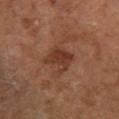This lesion was catalogued during total-body skin photography and was not selected for biopsy. The total-body-photography lesion software estimated an eccentricity of roughly 0.55 and a shape-asymmetry score of about 0.3 (0 = symmetric). It also reported border irregularity of about 3.5 on a 0–10 scale, internal color variation of about 2.5 on a 0–10 scale, and peripheral color asymmetry of about 1. A region of skin cropped from a whole-body photographic capture, roughly 15 mm wide. On the left lower leg. Measured at roughly 3.5 mm in maximum diameter. A female patient, aged 63 to 67.